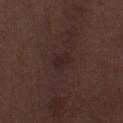About 2.5 mm across. Captured under white-light illumination. Located on the right thigh. A roughly 15 mm field-of-view crop from a total-body skin photograph. A male subject aged around 70.